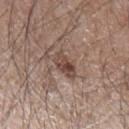The lesion was photographed on a routine skin check and not biopsied; there is no pathology result.
Cropped from a total-body skin-imaging series; the visible field is about 15 mm.
Approximately 3.5 mm at its widest.
The lesion-visualizer software estimated a lesion area of about 6.5 mm² and a shape eccentricity near 0.8. And it measured a lesion color around L≈46 a*≈17 b*≈24 in CIELAB and about 10 CIELAB-L* units darker than the surrounding skin. It also reported border irregularity of about 2.5 on a 0–10 scale.
Captured under white-light illumination.
A male subject, aged 63–67.
The lesion is on the left forearm.